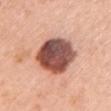Clinical impression: No biopsy was performed on this lesion — it was imaged during a full skin examination and was not determined to be concerning.